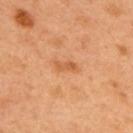<case>
  <biopsy_status>not biopsied; imaged during a skin examination</biopsy_status>
  <site>back</site>
  <lesion_size>
    <long_diameter_mm_approx>2.5</long_diameter_mm_approx>
  </lesion_size>
  <image>
    <source>total-body photography crop</source>
    <field_of_view_mm>15</field_of_view_mm>
  </image>
  <patient>
    <sex>male</sex>
    <age_approx>50</age_approx>
  </patient>
</case>